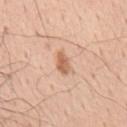Impression: This lesion was catalogued during total-body skin photography and was not selected for biopsy. Clinical summary: About 3 mm across. The lesion is on the upper back. The subject is a male in their mid- to late 50s. Imaged with white-light lighting. A close-up tile cropped from a whole-body skin photograph, about 15 mm across.